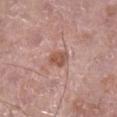* workup: catalogued during a skin exam; not biopsied
* acquisition: 15 mm crop, total-body photography
* automated lesion analysis: a footprint of about 5 mm², a shape eccentricity near 0.7, and a symmetry-axis asymmetry near 0.15; a lesion color around L≈54 a*≈22 b*≈28 in CIELAB and about 10 CIELAB-L* units darker than the surrounding skin
* patient: male, aged around 70
* diameter: about 3 mm
* body site: the right lower leg